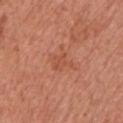  biopsy_status: not biopsied; imaged during a skin examination
  lesion_size:
    long_diameter_mm_approx: 2.5
  patient:
    sex: male
    age_approx: 65
  image:
    source: total-body photography crop
    field_of_view_mm: 15
  lighting: white-light
  automated_metrics:
    cielab_L: 52
    cielab_a: 28
    cielab_b: 34
    vs_skin_darker_L: 6.0
    vs_skin_contrast_norm: 4.5
  site: left upper arm